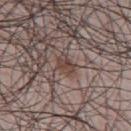biopsy_status: not biopsied; imaged during a skin examination
lesion_size:
  long_diameter_mm_approx: 3.0
site: chest
image:
  source: total-body photography crop
  field_of_view_mm: 15
patient:
  sex: male
  age_approx: 45
lighting: white-light